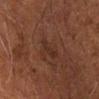This lesion was catalogued during total-body skin photography and was not selected for biopsy.
This is a cross-polarized tile.
Located on the left arm.
The patient is a male aged approximately 60.
The lesion's longest dimension is about 3 mm.
Automated image analysis of the tile measured a footprint of about 3 mm², an outline eccentricity of about 0.9 (0 = round, 1 = elongated), and a symmetry-axis asymmetry near 0.5.
A 15 mm close-up tile from a total-body photography series done for melanoma screening.